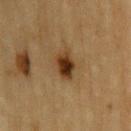follow-up — catalogued during a skin exam; not biopsied | image — ~15 mm crop, total-body skin-cancer survey | tile lighting — cross-polarized illumination | site — the left upper arm | subject — male, aged approximately 85 or older | automated lesion analysis — a mean CIELAB color near L≈30 a*≈17 b*≈30; an automated nevus-likeness rating near 100 out of 100 and a lesion-detection confidence of about 100/100 | lesion diameter — about 3 mm.